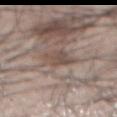Imaged during a routine full-body skin examination; the lesion was not biopsied and no histopathology is available. A roughly 15 mm field-of-view crop from a total-body skin photograph. Automated image analysis of the tile measured an area of roughly 6 mm² and an outline eccentricity of about 0.55 (0 = round, 1 = elongated). It also reported a mean CIELAB color near L≈49 a*≈14 b*≈22 and about 8 CIELAB-L* units darker than the surrounding skin. The software also gave an automated nevus-likeness rating near 0 out of 100 and lesion-presence confidence of about 55/100. Measured at roughly 3 mm in maximum diameter. The subject is a male aged 43–47. The lesion is located on the abdomen. This is a white-light tile.